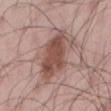{
  "biopsy_status": "not biopsied; imaged during a skin examination",
  "patient": {
    "sex": "male",
    "age_approx": 45
  },
  "site": "back",
  "lighting": "white-light",
  "image": {
    "source": "total-body photography crop",
    "field_of_view_mm": 15
  }
}